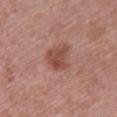Clinical impression:
No biopsy was performed on this lesion — it was imaged during a full skin examination and was not determined to be concerning.
Image and clinical context:
The tile uses white-light illumination. The lesion is on the back. An algorithmic analysis of the crop reported a lesion area of about 7.5 mm², an outline eccentricity of about 0.55 (0 = round, 1 = elongated), and a shape-asymmetry score of about 0.3 (0 = symmetric). The analysis additionally found a mean CIELAB color near L≈48 a*≈24 b*≈26. And it measured a classifier nevus-likeness of about 55/100 and a lesion-detection confidence of about 100/100. A 15 mm close-up tile from a total-body photography series done for melanoma screening. A female subject, aged 63–67.